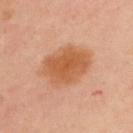Part of a total-body skin-imaging series; this lesion was reviewed on a skin check and was not flagged for biopsy.